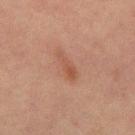notes: total-body-photography surveillance lesion; no biopsy
image: 15 mm crop, total-body photography
size: about 4 mm
anatomic site: the front of the torso
illumination: cross-polarized
subject: male, aged approximately 65